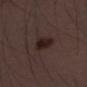Part of a total-body skin-imaging series; this lesion was reviewed on a skin check and was not flagged for biopsy. A 15 mm crop from a total-body photograph taken for skin-cancer surveillance. The total-body-photography lesion software estimated a border-irregularity index near 2/10, a color-variation rating of about 3/10, and peripheral color asymmetry of about 1. It also reported a classifier nevus-likeness of about 85/100 and a lesion-detection confidence of about 100/100. The tile uses white-light illumination. On the right thigh. A male subject approximately 30 years of age. The lesion's longest dimension is about 4 mm.